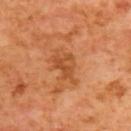Part of a total-body skin-imaging series; this lesion was reviewed on a skin check and was not flagged for biopsy. The lesion is on the upper back. A close-up tile cropped from a whole-body skin photograph, about 15 mm across. A male subject in their mid-60s. The recorded lesion diameter is about 4 mm. The tile uses cross-polarized illumination. Automated tile analysis of the lesion measured a lesion color around L≈49 a*≈27 b*≈41 in CIELAB, a lesion–skin lightness drop of about 8, and a normalized lesion–skin contrast near 6. The analysis additionally found border irregularity of about 5 on a 0–10 scale, a within-lesion color-variation index near 3.5/10, and peripheral color asymmetry of about 1.